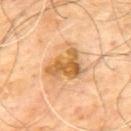| feature | finding |
|---|---|
| workup | no biopsy performed (imaged during a skin exam) |
| lesion size | ≈5 mm |
| imaging modality | ~15 mm tile from a whole-body skin photo |
| anatomic site | the mid back |
| subject | male, aged approximately 65 |
| image-analysis metrics | an area of roughly 13 mm²; a mean CIELAB color near L≈61 a*≈20 b*≈43, a lesion–skin lightness drop of about 12, and a normalized border contrast of about 8.5; border irregularity of about 5 on a 0–10 scale, a color-variation rating of about 6/10, and a peripheral color-asymmetry measure near 2; a detector confidence of about 100 out of 100 that the crop contains a lesion |